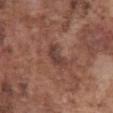workup: imaged on a skin check; not biopsied
acquisition: ~15 mm crop, total-body skin-cancer survey
tile lighting: white-light illumination
size: about 3.5 mm
body site: the abdomen
subject: male, roughly 75 years of age